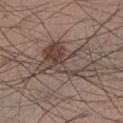The lesion is located on the left lower leg.
Captured under white-light illumination.
A 15 mm close-up tile from a total-body photography series done for melanoma screening.
A male subject about 35 years old.
Longest diameter approximately 8 mm.
An algorithmic analysis of the crop reported an area of roughly 17 mm² and an eccentricity of roughly 0.8. And it measured a classifier nevus-likeness of about 30/100.